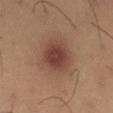Impression: Part of a total-body skin-imaging series; this lesion was reviewed on a skin check and was not flagged for biopsy. Context: The lesion is located on the leg. This image is a 15 mm lesion crop taken from a total-body photograph. Captured under cross-polarized illumination. The patient is a male in their mid-30s. The lesion's longest dimension is about 4 mm.